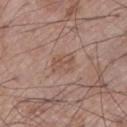Q: Was a biopsy performed?
A: total-body-photography surveillance lesion; no biopsy
Q: How large is the lesion?
A: about 3 mm
Q: Patient demographics?
A: male, approximately 70 years of age
Q: Automated lesion metrics?
A: an area of roughly 5.5 mm² and a shape-asymmetry score of about 0.25 (0 = symmetric); internal color variation of about 2.5 on a 0–10 scale; a classifier nevus-likeness of about 0/100 and a detector confidence of about 100 out of 100 that the crop contains a lesion
Q: Where on the body is the lesion?
A: the left lower leg
Q: What kind of image is this?
A: ~15 mm crop, total-body skin-cancer survey